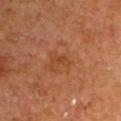Findings:
– follow-up: no biopsy performed (imaged during a skin exam)
– TBP lesion metrics: an average lesion color of about L≈42 a*≈26 b*≈35 (CIELAB), a lesion–skin lightness drop of about 6, and a normalized border contrast of about 5.5
– tile lighting: cross-polarized illumination
– image source: ~15 mm crop, total-body skin-cancer survey
– location: the left upper arm
– patient: male, aged around 65
– size: ≈2.5 mm